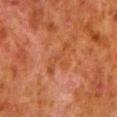Recorded during total-body skin imaging; not selected for excision or biopsy.
The lesion is on the left lower leg.
The lesion-visualizer software estimated an area of roughly 5 mm², a shape eccentricity near 0.9, and a shape-asymmetry score of about 0.55 (0 = symmetric). It also reported a within-lesion color-variation index near 0/10.
Longest diameter approximately 4.5 mm.
A 15 mm close-up tile from a total-body photography series done for melanoma screening.
This is a cross-polarized tile.
The subject is a male aged 78 to 82.